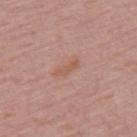workup: catalogued during a skin exam; not biopsied
diameter: ≈3 mm
subject: female, in their 50s
automated metrics: an outline eccentricity of about 0.95 (0 = round, 1 = elongated) and a shape-asymmetry score of about 0.35 (0 = symmetric); a border-irregularity rating of about 4/10 and internal color variation of about 0 on a 0–10 scale; lesion-presence confidence of about 100/100
lighting: white-light illumination
location: the back
imaging modality: ~15 mm crop, total-body skin-cancer survey